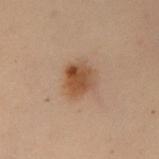Part of a total-body skin-imaging series; this lesion was reviewed on a skin check and was not flagged for biopsy.
A female subject in their 50s.
Automated tile analysis of the lesion measured an area of roughly 9.5 mm² and two-axis asymmetry of about 0.2. The analysis additionally found a border-irregularity index near 2/10 and a within-lesion color-variation index near 5/10. The analysis additionally found a lesion-detection confidence of about 100/100.
This is a cross-polarized tile.
Located on the arm.
The lesion's longest dimension is about 4 mm.
A 15 mm close-up tile from a total-body photography series done for melanoma screening.